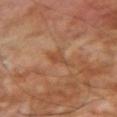On the arm. A 15 mm crop from a total-body photograph taken for skin-cancer surveillance. The lesion-visualizer software estimated a lesion area of about 3 mm² and a shape eccentricity near 0.8. And it measured lesion-presence confidence of about 95/100. Imaged with cross-polarized lighting. A male subject about 70 years old. About 2.5 mm across.